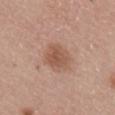Q: What did automated image analysis measure?
A: a footprint of about 10 mm², a shape eccentricity near 0.7, and a shape-asymmetry score of about 0.2 (0 = symmetric); a mean CIELAB color near L≈54 a*≈20 b*≈29, a lesion–skin lightness drop of about 9, and a normalized border contrast of about 6.5
Q: What is the imaging modality?
A: ~15 mm tile from a whole-body skin photo
Q: Patient demographics?
A: female, aged 63 to 67
Q: What lighting was used for the tile?
A: white-light illumination
Q: Where on the body is the lesion?
A: the upper back